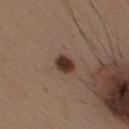- follow-up — imaged on a skin check; not biopsied
- image source — ~15 mm crop, total-body skin-cancer survey
- patient — male, in their 40s
- site — the mid back
- diameter — about 2.5 mm